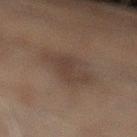About 6.5 mm across. Located on the left lower leg. A male subject, aged 58–62. Automated tile analysis of the lesion measured a mean CIELAB color near L≈29 a*≈10 b*≈18, a lesion–skin lightness drop of about 5, and a normalized lesion–skin contrast near 5.5. The software also gave an automated nevus-likeness rating near 5 out of 100 and a lesion-detection confidence of about 100/100. This image is a 15 mm lesion crop taken from a total-body photograph. The tile uses cross-polarized illumination.